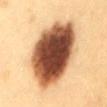Q: Was a biopsy performed?
A: total-body-photography surveillance lesion; no biopsy
Q: What is the anatomic site?
A: the chest
Q: How was the tile lit?
A: cross-polarized
Q: Who is the patient?
A: female, aged around 30
Q: What is the lesion's diameter?
A: ≈10.5 mm
Q: What is the imaging modality?
A: total-body-photography crop, ~15 mm field of view